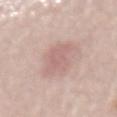No biopsy was performed on this lesion — it was imaged during a full skin examination and was not determined to be concerning. Captured under white-light illumination. A close-up tile cropped from a whole-body skin photograph, about 15 mm across. An algorithmic analysis of the crop reported an area of roughly 10 mm² and a symmetry-axis asymmetry near 0.3. The software also gave an average lesion color of about L≈63 a*≈19 b*≈22 (CIELAB). The analysis additionally found a classifier nevus-likeness of about 5/100 and a detector confidence of about 100 out of 100 that the crop contains a lesion. The lesion is located on the mid back. A female patient in their mid- to late 60s. Measured at roughly 5.5 mm in maximum diameter.